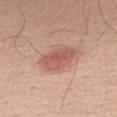Q: Is there a histopathology result?
A: catalogued during a skin exam; not biopsied
Q: What is the imaging modality?
A: ~15 mm crop, total-body skin-cancer survey
Q: Who is the patient?
A: male, in their 70s
Q: What is the anatomic site?
A: the right thigh
Q: Illumination type?
A: white-light illumination